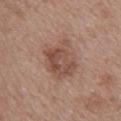Case summary:
- notes: total-body-photography surveillance lesion; no biopsy
- location: the upper back
- image source: ~15 mm tile from a whole-body skin photo
- subject: female, aged around 50